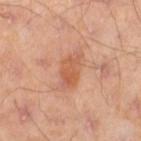acquisition: total-body-photography crop, ~15 mm field of view | patient: male, aged around 45 | body site: the left thigh | lighting: cross-polarized illumination.